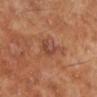Q: Was a biopsy performed?
A: imaged on a skin check; not biopsied
Q: Lesion size?
A: ≈3.5 mm
Q: Lesion location?
A: the left lower leg
Q: How was the tile lit?
A: cross-polarized illumination
Q: What are the patient's age and sex?
A: male, about 70 years old
Q: What did automated image analysis measure?
A: an average lesion color of about L≈44 a*≈23 b*≈29 (CIELAB), roughly 7 lightness units darker than nearby skin, and a normalized lesion–skin contrast near 6; a border-irregularity rating of about 5/10, internal color variation of about 2.5 on a 0–10 scale, and radial color variation of about 0.5; a nevus-likeness score of about 0/100 and a lesion-detection confidence of about 100/100
Q: What kind of image is this?
A: ~15 mm crop, total-body skin-cancer survey